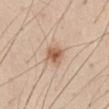  biopsy_status: not biopsied; imaged during a skin examination
  patient:
    sex: male
    age_approx: 45
  automated_metrics:
    cielab_L: 59
    cielab_a: 20
    cielab_b: 31
    vs_skin_darker_L: 12.0
    vs_skin_contrast_norm: 8.0
  lighting: white-light
  site: abdomen
  lesion_size:
    long_diameter_mm_approx: 2.5
  image:
    source: total-body photography crop
    field_of_view_mm: 15